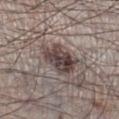Clinical impression: Recorded during total-body skin imaging; not selected for excision or biopsy. Image and clinical context: A male patient approximately 60 years of age. A 15 mm close-up tile from a total-body photography series done for melanoma screening. Measured at roughly 5 mm in maximum diameter. On the right lower leg.